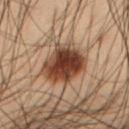Imaged during a routine full-body skin examination; the lesion was not biopsied and no histopathology is available. The patient is a male about 55 years old. The lesion is located on the abdomen. This is a cross-polarized tile. Approximately 5 mm at its widest. Cropped from a total-body skin-imaging series; the visible field is about 15 mm. Automated image analysis of the tile measured an area of roughly 15 mm², an eccentricity of roughly 0.65, and a symmetry-axis asymmetry near 0.15. The analysis additionally found a mean CIELAB color near L≈31 a*≈18 b*≈25. The software also gave a color-variation rating of about 5.5/10 and peripheral color asymmetry of about 2. The analysis additionally found lesion-presence confidence of about 100/100.